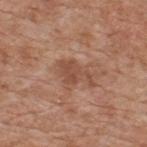{"biopsy_status": "not biopsied; imaged during a skin examination", "patient": {"sex": "male", "age_approx": 60}, "site": "back", "lighting": "white-light", "lesion_size": {"long_diameter_mm_approx": 4.5}, "image": {"source": "total-body photography crop", "field_of_view_mm": 15}}